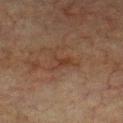This image is a 15 mm lesion crop taken from a total-body photograph. The lesion-visualizer software estimated border irregularity of about 6.5 on a 0–10 scale, a color-variation rating of about 3/10, and a peripheral color-asymmetry measure near 1. On the chest. The tile uses cross-polarized illumination. A male subject in their mid-70s.